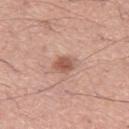Recorded during total-body skin imaging; not selected for excision or biopsy. The lesion-visualizer software estimated a footprint of about 4.5 mm², a shape eccentricity near 0.6, and two-axis asymmetry of about 0.35. The analysis additionally found a mean CIELAB color near L≈56 a*≈23 b*≈28, about 12 CIELAB-L* units darker than the surrounding skin, and a normalized border contrast of about 8. The software also gave border irregularity of about 3 on a 0–10 scale, internal color variation of about 3.5 on a 0–10 scale, and a peripheral color-asymmetry measure near 1. And it measured a nevus-likeness score of about 90/100 and a detector confidence of about 100 out of 100 that the crop contains a lesion. This is a white-light tile. A roughly 15 mm field-of-view crop from a total-body skin photograph. Approximately 2.5 mm at its widest. The patient is a male about 55 years old.